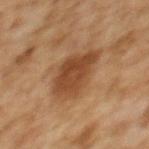size: ~5.5 mm (longest diameter) | image: ~15 mm tile from a whole-body skin photo | patient: female, aged approximately 60 | site: the upper back | illumination: cross-polarized illumination.